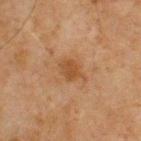workup = imaged on a skin check; not biopsied | acquisition = ~15 mm crop, total-body skin-cancer survey | location = the front of the torso | illumination = cross-polarized illumination | patient = male, roughly 65 years of age | lesion size = about 2.5 mm.